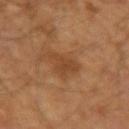Part of a total-body skin-imaging series; this lesion was reviewed on a skin check and was not flagged for biopsy. The recorded lesion diameter is about 3.5 mm. A male subject, in their mid-60s. An algorithmic analysis of the crop reported an average lesion color of about L≈42 a*≈21 b*≈34 (CIELAB) and a normalized lesion–skin contrast near 6. And it measured border irregularity of about 5.5 on a 0–10 scale, internal color variation of about 2 on a 0–10 scale, and a peripheral color-asymmetry measure near 0.5. The analysis additionally found an automated nevus-likeness rating near 5 out of 100 and a lesion-detection confidence of about 100/100. The lesion is located on the left upper arm. Captured under cross-polarized illumination. A 15 mm crop from a total-body photograph taken for skin-cancer surveillance.